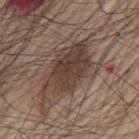Impression:
The lesion was tiled from a total-body skin photograph and was not biopsied.
Acquisition and patient details:
The lesion is on the mid back. A male subject aged 68 to 72. Cropped from a total-body skin-imaging series; the visible field is about 15 mm.